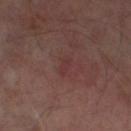Case summary:
- subject: male, approximately 65 years of age
- location: the left thigh
- lighting: cross-polarized
- lesion size: ≈2.5 mm
- image source: ~15 mm crop, total-body skin-cancer survey